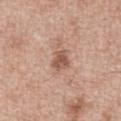This lesion was catalogued during total-body skin photography and was not selected for biopsy. The recorded lesion diameter is about 2.5 mm. This is a white-light tile. A 15 mm close-up tile from a total-body photography series done for melanoma screening. The patient is a male about 50 years old. From the front of the torso.